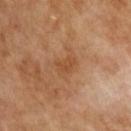Impression:
The lesion was tiled from a total-body skin photograph and was not biopsied.
Clinical summary:
A 15 mm crop from a total-body photograph taken for skin-cancer surveillance. The lesion's longest dimension is about 3 mm. A male patient about 65 years old.